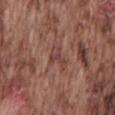Part of a total-body skin-imaging series; this lesion was reviewed on a skin check and was not flagged for biopsy. A male subject in their mid-70s. Measured at roughly 2.5 mm in maximum diameter. Imaged with white-light lighting. From the mid back. A 15 mm crop from a total-body photograph taken for skin-cancer surveillance. Automated image analysis of the tile measured border irregularity of about 6 on a 0–10 scale, a color-variation rating of about 2/10, and peripheral color asymmetry of about 0.5.